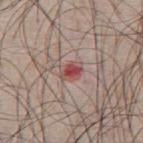Q: Is there a histopathology result?
A: no biopsy performed (imaged during a skin exam)
Q: What did automated image analysis measure?
A: a mean CIELAB color near L≈49 a*≈27 b*≈23, a lesion–skin lightness drop of about 11, and a lesion-to-skin contrast of about 8.5 (normalized; higher = more distinct); an automated nevus-likeness rating near 0 out of 100 and lesion-presence confidence of about 100/100
Q: Who is the patient?
A: male, aged approximately 70
Q: What is the lesion's diameter?
A: ~3 mm (longest diameter)
Q: How was the tile lit?
A: white-light illumination
Q: Lesion location?
A: the front of the torso
Q: What is the imaging modality?
A: ~15 mm crop, total-body skin-cancer survey